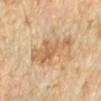<case>
  <biopsy_status>not biopsied; imaged during a skin examination</biopsy_status>
  <lighting>cross-polarized</lighting>
  <image>
    <source>total-body photography crop</source>
    <field_of_view_mm>15</field_of_view_mm>
  </image>
  <site>arm</site>
  <patient>
    <sex>female</sex>
    <age_approx>45</age_approx>
  </patient>
  <automated_metrics>
    <border_irregularity_0_10>6.0</border_irregularity_0_10>
    <lesion_detection_confidence_0_100>100</lesion_detection_confidence_0_100>
  </automated_metrics>
</case>